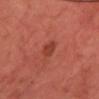The lesion was photographed on a routine skin check and not biopsied; there is no pathology result. On the head or neck. A male subject approximately 70 years of age. A close-up tile cropped from a whole-body skin photograph, about 15 mm across. Automated image analysis of the tile measured an area of roughly 3.5 mm² and a shape eccentricity near 0.75.